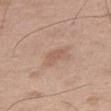A 15 mm close-up tile from a total-body photography series done for melanoma screening.
Automated image analysis of the tile measured a lesion color around L≈58 a*≈19 b*≈29 in CIELAB and roughly 7 lightness units darker than nearby skin. The software also gave a lesion-detection confidence of about 100/100.
From the right thigh.
This is a white-light tile.
A male subject, aged approximately 50.